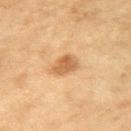Impression: The lesion was tiled from a total-body skin photograph and was not biopsied. Image and clinical context: A female patient, approximately 60 years of age. The tile uses cross-polarized illumination. Cropped from a whole-body photographic skin survey; the tile spans about 15 mm. The lesion is located on the left upper arm.